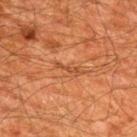workup: imaged on a skin check; not biopsied | patient: male, in their 60s | diameter: ≈3 mm | lighting: cross-polarized illumination | acquisition: total-body-photography crop, ~15 mm field of view | body site: the upper back.